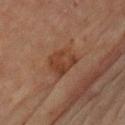* biopsy status: no biopsy performed (imaged during a skin exam)
* tile lighting: cross-polarized
* image source: 15 mm crop, total-body photography
* site: the left upper arm
* diameter: ~3.5 mm (longest diameter)
* subject: female, about 80 years old